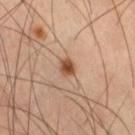Imaged during a routine full-body skin examination; the lesion was not biopsied and no histopathology is available. The lesion is located on the right thigh. A male subject in their 60s. About 2.5 mm across. An algorithmic analysis of the crop reported an area of roughly 3.5 mm², a shape eccentricity near 0.6, and a shape-asymmetry score of about 0.25 (0 = symmetric). And it measured an average lesion color of about L≈48 a*≈21 b*≈32 (CIELAB) and roughly 13 lightness units darker than nearby skin. The analysis additionally found a border-irregularity rating of about 2/10 and peripheral color asymmetry of about 0.5. And it measured a lesion-detection confidence of about 100/100. A 15 mm close-up extracted from a 3D total-body photography capture. Imaged with cross-polarized lighting.